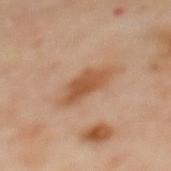Part of a total-body skin-imaging series; this lesion was reviewed on a skin check and was not flagged for biopsy. About 6 mm across. The lesion is on the upper back. The total-body-photography lesion software estimated a border-irregularity index near 3.5/10, a color-variation rating of about 3/10, and radial color variation of about 1. The analysis additionally found an automated nevus-likeness rating near 80 out of 100. A region of skin cropped from a whole-body photographic capture, roughly 15 mm wide. A female patient aged 58–62. This is a cross-polarized tile.